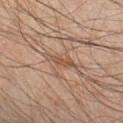Q: What did automated image analysis measure?
A: an automated nevus-likeness rating near 0 out of 100 and a lesion-detection confidence of about 90/100
Q: Who is the patient?
A: male, roughly 45 years of age
Q: How was the tile lit?
A: cross-polarized
Q: How was this image acquired?
A: 15 mm crop, total-body photography
Q: Where on the body is the lesion?
A: the left forearm
Q: What is the lesion's diameter?
A: ~3.5 mm (longest diameter)